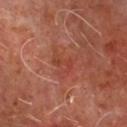illumination: cross-polarized illumination
subject: male, aged 63 to 67
anatomic site: the chest
image source: ~15 mm crop, total-body skin-cancer survey
automated lesion analysis: a lesion area of about 2.5 mm², a shape eccentricity near 0.9, and a symmetry-axis asymmetry near 0.45; a normalized border contrast of about 5; a border-irregularity rating of about 5.5/10; an automated nevus-likeness rating near 0 out of 100 and a lesion-detection confidence of about 100/100